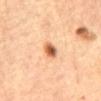Context:
The subject is a female aged around 65. The lesion's longest dimension is about 3 mm. The total-body-photography lesion software estimated a color-variation rating of about 5/10 and peripheral color asymmetry of about 1.5. The lesion is located on the chest. Captured under cross-polarized illumination. Cropped from a total-body skin-imaging series; the visible field is about 15 mm.